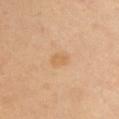image source: ~15 mm crop, total-body skin-cancer survey
automated lesion analysis: a footprint of about 4.5 mm², an outline eccentricity of about 0.7 (0 = round, 1 = elongated), and a symmetry-axis asymmetry near 0.2; a lesion color around L≈62 a*≈18 b*≈38 in CIELAB, roughly 6 lightness units darker than nearby skin, and a normalized lesion–skin contrast near 5; border irregularity of about 2 on a 0–10 scale and a color-variation rating of about 1.5/10; an automated nevus-likeness rating near 5 out of 100 and a detector confidence of about 100 out of 100 that the crop contains a lesion
lesion diameter: ~2.5 mm (longest diameter)
illumination: cross-polarized
subject: female, in their mid- to late 30s
site: the head or neck